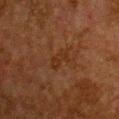Clinical impression: Imaged during a routine full-body skin examination; the lesion was not biopsied and no histopathology is available. Image and clinical context: A 15 mm close-up tile from a total-body photography series done for melanoma screening. The tile uses cross-polarized illumination. From the chest. Approximately 3 mm at its widest. The patient is a female aged around 50. An algorithmic analysis of the crop reported a lesion color around L≈25 a*≈18 b*≈27 in CIELAB, about 4 CIELAB-L* units darker than the surrounding skin, and a lesion-to-skin contrast of about 5.5 (normalized; higher = more distinct). It also reported a border-irregularity rating of about 3.5/10, internal color variation of about 1 on a 0–10 scale, and radial color variation of about 0.5.